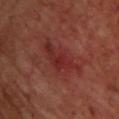Imaged during a routine full-body skin examination; the lesion was not biopsied and no histopathology is available. The lesion is located on the upper back. A 15 mm close-up tile from a total-body photography series done for melanoma screening. A male patient, about 70 years old. Captured under cross-polarized illumination. The total-body-photography lesion software estimated a shape-asymmetry score of about 0.35 (0 = symmetric). The analysis additionally found an average lesion color of about L≈29 a*≈28 b*≈23 (CIELAB), roughly 7 lightness units darker than nearby skin, and a normalized border contrast of about 6.5. The software also gave a nevus-likeness score of about 0/100 and lesion-presence confidence of about 95/100. Measured at roughly 6.5 mm in maximum diameter.